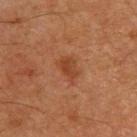Part of a total-body skin-imaging series; this lesion was reviewed on a skin check and was not flagged for biopsy.